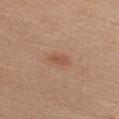A roughly 15 mm field-of-view crop from a total-body skin photograph. From the chest. The tile uses white-light illumination. A female patient, approximately 45 years of age. Automated image analysis of the tile measured an area of roughly 3 mm², an outline eccentricity of about 0.85 (0 = round, 1 = elongated), and two-axis asymmetry of about 0.35. It also reported a lesion color around L≈52 a*≈23 b*≈31 in CIELAB, roughly 8 lightness units darker than nearby skin, and a normalized border contrast of about 6. It also reported a border-irregularity rating of about 3.5/10, a within-lesion color-variation index near 1.5/10, and peripheral color asymmetry of about 0.5. About 2.5 mm across.